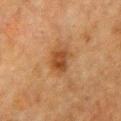| feature | finding |
|---|---|
| follow-up | catalogued during a skin exam; not biopsied |
| subject | female, approximately 55 years of age |
| site | the chest |
| automated metrics | a border-irregularity index near 2/10, internal color variation of about 3 on a 0–10 scale, and radial color variation of about 1; a lesion-detection confidence of about 100/100 |
| acquisition | ~15 mm tile from a whole-body skin photo |